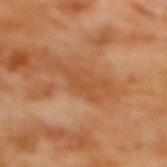<case>
<biopsy_status>not biopsied; imaged during a skin examination</biopsy_status>
<site>mid back</site>
<lesion_size>
  <long_diameter_mm_approx>5.0</long_diameter_mm_approx>
</lesion_size>
<patient>
  <sex>female</sex>
  <age_approx>55</age_approx>
</patient>
<image>
  <source>total-body photography crop</source>
  <field_of_view_mm>15</field_of_view_mm>
</image>
<lighting>cross-polarized</lighting>
</case>